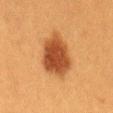| key | value |
|---|---|
| workup | no biopsy performed (imaged during a skin exam) |
| body site | the back |
| illumination | cross-polarized |
| patient | female, aged 38 to 42 |
| imaging modality | ~15 mm tile from a whole-body skin photo |